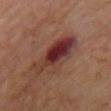Impression: Part of a total-body skin-imaging series; this lesion was reviewed on a skin check and was not flagged for biopsy. Background: A male patient aged approximately 70. The lesion is on the chest. A lesion tile, about 15 mm wide, cut from a 3D total-body photograph. Automated tile analysis of the lesion measured a footprint of about 17 mm², an outline eccentricity of about 0.85 (0 = round, 1 = elongated), and a symmetry-axis asymmetry near 0.3. The software also gave a lesion color around L≈34 a*≈23 b*≈23 in CIELAB, a lesion–skin lightness drop of about 12, and a normalized lesion–skin contrast near 10.5. And it measured a border-irregularity index near 4/10 and radial color variation of about 3.5. The analysis additionally found a classifier nevus-likeness of about 0/100 and a lesion-detection confidence of about 100/100. The tile uses cross-polarized illumination. Approximately 6.5 mm at its widest.